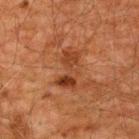Assessment: The lesion was tiled from a total-body skin photograph and was not biopsied. Background: Cropped from a total-body skin-imaging series; the visible field is about 15 mm. A male patient, aged approximately 60. Measured at roughly 4.5 mm in maximum diameter. Located on the upper back. The tile uses cross-polarized illumination.